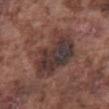biopsy_status: not biopsied; imaged during a skin examination
lighting: white-light
site: abdomen
automated_metrics:
  area_mm2_approx: 23.0
  eccentricity: 0.7
  shape_asymmetry: 0.3
  nevus_likeness_0_100: 0
lesion_size:
  long_diameter_mm_approx: 6.5
image:
  source: total-body photography crop
  field_of_view_mm: 15
patient:
  sex: male
  age_approx: 75